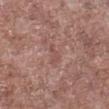biopsy status: no biopsy performed (imaged during a skin exam); illumination: white-light illumination; subject: male, roughly 55 years of age; image source: ~15 mm tile from a whole-body skin photo; location: the right lower leg; lesion size: about 3 mm; automated lesion analysis: a lesion area of about 2.5 mm², an outline eccentricity of about 0.9 (0 = round, 1 = elongated), and a symmetry-axis asymmetry near 0.5.